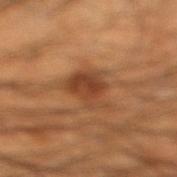Recorded during total-body skin imaging; not selected for excision or biopsy.
A 15 mm crop from a total-body photograph taken for skin-cancer surveillance.
A male subject, approximately 45 years of age.
From the right lower leg.